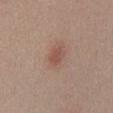Imaged during a routine full-body skin examination; the lesion was not biopsied and no histopathology is available. Cropped from a total-body skin-imaging series; the visible field is about 15 mm. An algorithmic analysis of the crop reported border irregularity of about 2 on a 0–10 scale, a color-variation rating of about 1.5/10, and peripheral color asymmetry of about 0.5. This is a white-light tile. A male patient in their mid- to late 50s. From the abdomen. Approximately 2.5 mm at its widest.